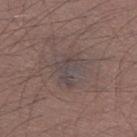Assessment: This lesion was catalogued during total-body skin photography and was not selected for biopsy. Background: From the left thigh. Measured at roughly 4.5 mm in maximum diameter. A roughly 15 mm field-of-view crop from a total-body skin photograph. Imaged with white-light lighting. The subject is a male approximately 35 years of age.